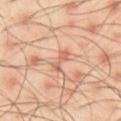Clinical impression:
Captured during whole-body skin photography for melanoma surveillance; the lesion was not biopsied.
Acquisition and patient details:
The lesion is on the chest. A roughly 15 mm field-of-view crop from a total-body skin photograph. A male patient in their mid-40s.